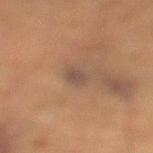subject: male, in their mid-80s or older | tile lighting: cross-polarized illumination | diameter: about 2.5 mm | location: the left lower leg | image source: 15 mm crop, total-body photography.